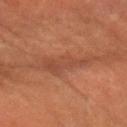{"biopsy_status": "not biopsied; imaged during a skin examination", "image": {"source": "total-body photography crop", "field_of_view_mm": 15}, "site": "right forearm", "lesion_size": {"long_diameter_mm_approx": 6.0}, "lighting": "cross-polarized", "patient": {"sex": "female", "age_approx": 80}}